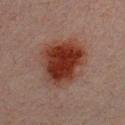<case>
<site>chest</site>
<patient>
  <sex>male</sex>
  <age_approx>30</age_approx>
</patient>
<automated_metrics>
  <area_mm2_approx>25.0</area_mm2_approx>
  <eccentricity>0.55</eccentricity>
  <shape_asymmetry>0.25</shape_asymmetry>
  <cielab_L>29</cielab_L>
  <cielab_a>22</cielab_a>
  <cielab_b>24</cielab_b>
  <vs_skin_darker_L>12.0</vs_skin_darker_L>
  <lesion_detection_confidence_0_100>100</lesion_detection_confidence_0_100>
</automated_metrics>
<lighting>cross-polarized</lighting>
<lesion_size>
  <long_diameter_mm_approx>6.5</long_diameter_mm_approx>
</lesion_size>
<image>
  <source>total-body photography crop</source>
  <field_of_view_mm>15</field_of_view_mm>
</image>
</case>